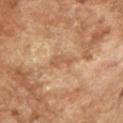Q: Was this lesion biopsied?
A: total-body-photography surveillance lesion; no biopsy
Q: What is the imaging modality?
A: ~15 mm crop, total-body skin-cancer survey
Q: What did automated image analysis measure?
A: an eccentricity of roughly 0.85 and a shape-asymmetry score of about 0.4 (0 = symmetric)
Q: Illumination type?
A: cross-polarized illumination
Q: Who is the patient?
A: male, in their mid- to late 60s
Q: What is the lesion's diameter?
A: ~3 mm (longest diameter)